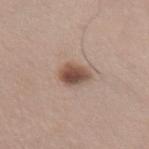Impression: The lesion was tiled from a total-body skin photograph and was not biopsied. Image and clinical context: A 15 mm crop from a total-body photograph taken for skin-cancer surveillance. This is a white-light tile. Located on the front of the torso. The recorded lesion diameter is about 3.5 mm. A female subject, aged 48 to 52.